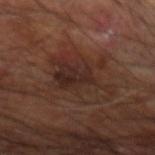This lesion was catalogued during total-body skin photography and was not selected for biopsy. A 15 mm close-up extracted from a 3D total-body photography capture. From the right arm. A male patient, aged around 60.